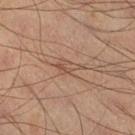This lesion was catalogued during total-body skin photography and was not selected for biopsy.
The lesion's longest dimension is about 3.5 mm.
A male patient, about 45 years old.
The tile uses cross-polarized illumination.
A 15 mm close-up extracted from a 3D total-body photography capture.
The lesion is located on the right lower leg.
The total-body-photography lesion software estimated a footprint of about 4.5 mm², an outline eccentricity of about 0.8 (0 = round, 1 = elongated), and a symmetry-axis asymmetry near 0.35. It also reported a nevus-likeness score of about 0/100 and a lesion-detection confidence of about 90/100.